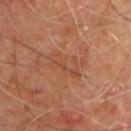| feature | finding |
|---|---|
| biopsy status | imaged on a skin check; not biopsied |
| TBP lesion metrics | a mean CIELAB color near L≈46 a*≈23 b*≈32 and a lesion-to-skin contrast of about 4.5 (normalized; higher = more distinct); a nevus-likeness score of about 0/100 and a lesion-detection confidence of about 100/100 |
| subject | male, aged 58 to 62 |
| illumination | cross-polarized |
| anatomic site | the upper back |
| image | ~15 mm crop, total-body skin-cancer survey |